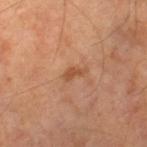Clinical impression:
Part of a total-body skin-imaging series; this lesion was reviewed on a skin check and was not flagged for biopsy.
Image and clinical context:
Measured at roughly 2.5 mm in maximum diameter. Automated image analysis of the tile measured a footprint of about 3 mm², an eccentricity of roughly 0.85, and two-axis asymmetry of about 0.45. It also reported a mean CIELAB color near L≈49 a*≈23 b*≈34 and a normalized lesion–skin contrast near 6.5. A male subject roughly 65 years of age. A close-up tile cropped from a whole-body skin photograph, about 15 mm across. The lesion is located on the right lower leg.